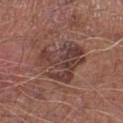workup: no biopsy performed (imaged during a skin exam) | location: the right lower leg | subject: male, aged approximately 65 | tile lighting: white-light | image source: ~15 mm crop, total-body skin-cancer survey | lesion diameter: ≈6.5 mm.